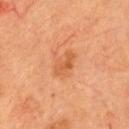The lesion was photographed on a routine skin check and not biopsied; there is no pathology result.
This is a cross-polarized tile.
The subject is a male in their 70s.
Approximately 3 mm at its widest.
The lesion is located on the chest.
A lesion tile, about 15 mm wide, cut from a 3D total-body photograph.